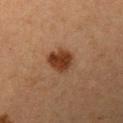| feature | finding |
|---|---|
| notes | no biopsy performed (imaged during a skin exam) |
| body site | the left upper arm |
| tile lighting | cross-polarized |
| imaging modality | 15 mm crop, total-body photography |
| patient | female, roughly 40 years of age |
| lesion diameter | about 3 mm |